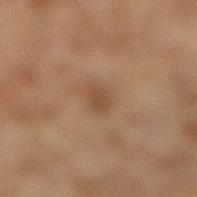Impression: This lesion was catalogued during total-body skin photography and was not selected for biopsy. Acquisition and patient details: The tile uses cross-polarized illumination. Automated tile analysis of the lesion measured an outline eccentricity of about 0.8 (0 = round, 1 = elongated) and two-axis asymmetry of about 0.2. It also reported a mean CIELAB color near L≈41 a*≈17 b*≈29 and a normalized border contrast of about 6. It also reported a border-irregularity index near 2/10, a within-lesion color-variation index near 1/10, and peripheral color asymmetry of about 0.5. From the right lower leg. Measured at roughly 2.5 mm in maximum diameter. A 15 mm close-up extracted from a 3D total-body photography capture. The subject is a female approximately 60 years of age.